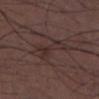TBP lesion metrics — a border-irregularity rating of about 6/10, a color-variation rating of about 3/10, and peripheral color asymmetry of about 1 | size — ~5.5 mm (longest diameter) | illumination — white-light | patient — male, approximately 30 years of age | image — ~15 mm tile from a whole-body skin photo | location — the chest.